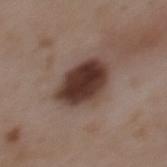Q: Is there a histopathology result?
A: no biopsy performed (imaged during a skin exam)
Q: Automated lesion metrics?
A: an area of roughly 16 mm² and a shape eccentricity near 0.65; a lesion color around L≈35 a*≈17 b*≈22 in CIELAB, a lesion–skin lightness drop of about 17, and a normalized border contrast of about 14; a color-variation rating of about 4.5/10 and peripheral color asymmetry of about 1.5; a nevus-likeness score of about 85/100
Q: How large is the lesion?
A: ≈5.5 mm
Q: How was the tile lit?
A: white-light illumination
Q: Patient demographics?
A: male, approximately 50 years of age
Q: Lesion location?
A: the mid back
Q: How was this image acquired?
A: 15 mm crop, total-body photography